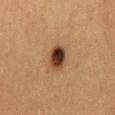Acquisition and patient details: A female patient, aged 38 to 42. The lesion is on the back. Cropped from a whole-body photographic skin survey; the tile spans about 15 mm. The tile uses cross-polarized illumination. The lesion-visualizer software estimated a lesion color around L≈34 a*≈16 b*≈27 in CIELAB, roughly 15 lightness units darker than nearby skin, and a normalized border contrast of about 13. And it measured border irregularity of about 1.5 on a 0–10 scale, a color-variation rating of about 8.5/10, and a peripheral color-asymmetry measure near 2.5. And it measured a nevus-likeness score of about 100/100.